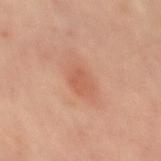biopsy_status: not biopsied; imaged during a skin examination
lighting: cross-polarized
patient:
  sex: male
  age_approx: 50
site: mid back
lesion_size:
  long_diameter_mm_approx: 4.5
image:
  source: total-body photography crop
  field_of_view_mm: 15